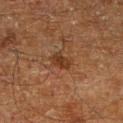{
  "biopsy_status": "not biopsied; imaged during a skin examination",
  "image": {
    "source": "total-body photography crop",
    "field_of_view_mm": 15
  },
  "automated_metrics": {
    "area_mm2_approx": 4.5,
    "eccentricity": 0.65,
    "shape_asymmetry": 0.25,
    "border_irregularity_0_10": 2.5,
    "color_variation_0_10": 2.0,
    "peripheral_color_asymmetry": 0.5,
    "nevus_likeness_0_100": 25,
    "lesion_detection_confidence_0_100": 100
  },
  "lighting": "cross-polarized",
  "patient": {
    "sex": "male",
    "age_approx": 60
  },
  "lesion_size": {
    "long_diameter_mm_approx": 2.5
  },
  "site": "right lower leg"
}